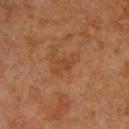biopsy status = total-body-photography surveillance lesion; no biopsy
patient = male, aged approximately 60
image-analysis metrics = about 6 CIELAB-L* units darker than the surrounding skin and a normalized border contrast of about 5
location = the upper back
diameter = ≈2.5 mm
tile lighting = cross-polarized illumination
imaging modality = total-body-photography crop, ~15 mm field of view